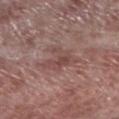This lesion was catalogued during total-body skin photography and was not selected for biopsy.
About 5 mm across.
A male patient aged 53 to 57.
The lesion is located on the left lower leg.
Cropped from a whole-body photographic skin survey; the tile spans about 15 mm.
This is a white-light tile.